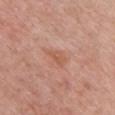Assessment:
No biopsy was performed on this lesion — it was imaged during a full skin examination and was not determined to be concerning.
Clinical summary:
Approximately 3 mm at its widest. A close-up tile cropped from a whole-body skin photograph, about 15 mm across. The lesion is on the chest. The tile uses white-light illumination. A female subject, in their 60s. Automated tile analysis of the lesion measured a lesion color around L≈57 a*≈24 b*≈32 in CIELAB, about 7 CIELAB-L* units darker than the surrounding skin, and a lesion-to-skin contrast of about 6 (normalized; higher = more distinct).